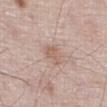Q: Was this lesion biopsied?
A: catalogued during a skin exam; not biopsied
Q: What is the imaging modality?
A: ~15 mm tile from a whole-body skin photo
Q: Lesion location?
A: the abdomen
Q: What are the patient's age and sex?
A: male, aged around 80